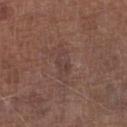This lesion was catalogued during total-body skin photography and was not selected for biopsy.
A male subject, in their mid-70s.
Located on the right lower leg.
A roughly 15 mm field-of-view crop from a total-body skin photograph.
Measured at roughly 3 mm in maximum diameter.
The total-body-photography lesion software estimated a lesion area of about 4.5 mm², an outline eccentricity of about 0.85 (0 = round, 1 = elongated), and two-axis asymmetry of about 0.3. The analysis additionally found a lesion–skin lightness drop of about 5 and a lesion-to-skin contrast of about 4.5 (normalized; higher = more distinct). And it measured a border-irregularity index near 3/10, a color-variation rating of about 2/10, and peripheral color asymmetry of about 0.5. The analysis additionally found a classifier nevus-likeness of about 0/100 and lesion-presence confidence of about 100/100.
This is a white-light tile.